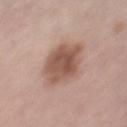Q: Was this lesion biopsied?
A: no biopsy performed (imaged during a skin exam)
Q: Patient demographics?
A: female, about 50 years old
Q: What is the lesion's diameter?
A: about 5 mm
Q: What lighting was used for the tile?
A: white-light illumination
Q: What is the anatomic site?
A: the chest
Q: What is the imaging modality?
A: total-body-photography crop, ~15 mm field of view
Q: Automated lesion metrics?
A: a footprint of about 15 mm²; an average lesion color of about L≈53 a*≈20 b*≈26 (CIELAB), a lesion–skin lightness drop of about 13, and a normalized lesion–skin contrast near 8.5; a border-irregularity index near 2/10, a within-lesion color-variation index near 4.5/10, and peripheral color asymmetry of about 1.5